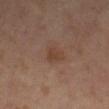biopsy status — imaged on a skin check; not biopsied
lighting — cross-polarized
subject — male, in their mid-60s
size — about 2.5 mm
acquisition — total-body-photography crop, ~15 mm field of view